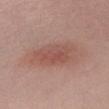{
  "biopsy_status": "not biopsied; imaged during a skin examination",
  "site": "abdomen",
  "image": {
    "source": "total-body photography crop",
    "field_of_view_mm": 15
  },
  "patient": {
    "sex": "female",
    "age_approx": 30
  }
}